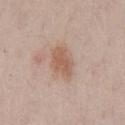The subject is a male aged around 35. The lesion's longest dimension is about 4 mm. Imaged with white-light lighting. This image is a 15 mm lesion crop taken from a total-body photograph. On the abdomen. The total-body-photography lesion software estimated a footprint of about 7 mm², an outline eccentricity of about 0.8 (0 = round, 1 = elongated), and two-axis asymmetry of about 0.2. It also reported a color-variation rating of about 2/10. The software also gave a classifier nevus-likeness of about 40/100 and a detector confidence of about 100 out of 100 that the crop contains a lesion.